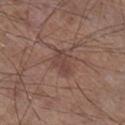Notes:
• biopsy status · total-body-photography surveillance lesion; no biopsy
• site · the right lower leg
• subject · male, approximately 60 years of age
• automated metrics · an average lesion color of about L≈42 a*≈19 b*≈23 (CIELAB), roughly 7 lightness units darker than nearby skin, and a lesion-to-skin contrast of about 6 (normalized; higher = more distinct); border irregularity of about 3.5 on a 0–10 scale and a color-variation rating of about 1.5/10
• tile lighting · white-light illumination
• acquisition · total-body-photography crop, ~15 mm field of view